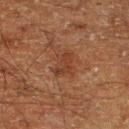- follow-up: no biopsy performed (imaged during a skin exam)
- subject: male, about 60 years old
- site: the leg
- acquisition: ~15 mm crop, total-body skin-cancer survey